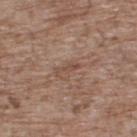A 15 mm crop from a total-body photograph taken for skin-cancer surveillance. A male patient, aged approximately 70. Approximately 3 mm at its widest. Located on the upper back. The tile uses white-light illumination.